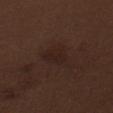No biopsy was performed on this lesion — it was imaged during a full skin examination and was not determined to be concerning.
A male patient, aged 68–72.
Imaged with white-light lighting.
The recorded lesion diameter is about 3.5 mm.
A roughly 15 mm field-of-view crop from a total-body skin photograph.
The total-body-photography lesion software estimated a lesion color around L≈21 a*≈15 b*≈19 in CIELAB, a lesion–skin lightness drop of about 4, and a lesion-to-skin contrast of about 5.5 (normalized; higher = more distinct). It also reported border irregularity of about 4 on a 0–10 scale and a within-lesion color-variation index near 2.5/10. It also reported lesion-presence confidence of about 100/100.
From the abdomen.